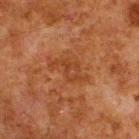Part of a total-body skin-imaging series; this lesion was reviewed on a skin check and was not flagged for biopsy.